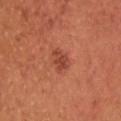follow-up: catalogued during a skin exam; not biopsied | image: total-body-photography crop, ~15 mm field of view | anatomic site: the head or neck | lighting: cross-polarized illumination | patient: female, aged 38 to 42 | lesion size: about 3 mm.